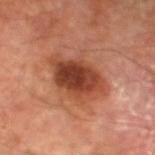This is a cross-polarized tile. The lesion is on the left lower leg. Longest diameter approximately 6.5 mm. An algorithmic analysis of the crop reported a classifier nevus-likeness of about 85/100. A male patient, aged approximately 70. A 15 mm crop from a total-body photograph taken for skin-cancer surveillance.